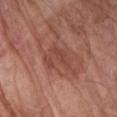Captured during whole-body skin photography for melanoma surveillance; the lesion was not biopsied.
A male subject roughly 80 years of age.
The tile uses white-light illumination.
This image is a 15 mm lesion crop taken from a total-body photograph.
Automated image analysis of the tile measured an area of roughly 7.5 mm², an eccentricity of roughly 0.75, and a symmetry-axis asymmetry near 0.5. The software also gave a lesion–skin lightness drop of about 7 and a normalized border contrast of about 5.5. It also reported a border-irregularity index near 8/10, internal color variation of about 1 on a 0–10 scale, and radial color variation of about 0.
On the right forearm.
Approximately 4 mm at its widest.